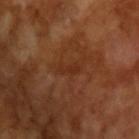No biopsy was performed on this lesion — it was imaged during a full skin examination and was not determined to be concerning. A male subject, aged approximately 65. The lesion's longest dimension is about 3 mm. Cropped from a whole-body photographic skin survey; the tile spans about 15 mm. The lesion-visualizer software estimated a mean CIELAB color near L≈29 a*≈24 b*≈31, about 5 CIELAB-L* units darker than the surrounding skin, and a normalized border contrast of about 5. And it measured border irregularity of about 3.5 on a 0–10 scale and a color-variation rating of about 0/10. It also reported lesion-presence confidence of about 100/100.